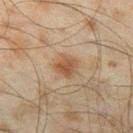Impression:
Recorded during total-body skin imaging; not selected for excision or biopsy.
Image and clinical context:
The recorded lesion diameter is about 2.5 mm. The lesion is located on the left thigh. Cropped from a whole-body photographic skin survey; the tile spans about 15 mm. This is a cross-polarized tile. Automated tile analysis of the lesion measured a footprint of about 5 mm², an eccentricity of roughly 0.4, and a symmetry-axis asymmetry near 0.3. The software also gave a mean CIELAB color near L≈42 a*≈16 b*≈28, about 8 CIELAB-L* units darker than the surrounding skin, and a normalized border contrast of about 7.5. The analysis additionally found lesion-presence confidence of about 100/100. A male subject roughly 45 years of age.